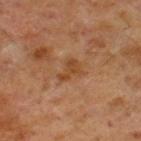No biopsy was performed on this lesion — it was imaged during a full skin examination and was not determined to be concerning. Cropped from a whole-body photographic skin survey; the tile spans about 15 mm. Captured under cross-polarized illumination. From the right lower leg. The subject is a male about 60 years old.